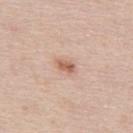notes = total-body-photography surveillance lesion; no biopsy | diameter = ≈2.5 mm | automated lesion analysis = peripheral color asymmetry of about 1; lesion-presence confidence of about 100/100 | image = 15 mm crop, total-body photography | subject = male, in their 40s | anatomic site = the upper back.